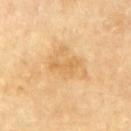Q: Patient demographics?
A: male, aged 83 or over
Q: What lighting was used for the tile?
A: cross-polarized illumination
Q: How large is the lesion?
A: ~3.5 mm (longest diameter)
Q: Where on the body is the lesion?
A: the back
Q: Automated lesion metrics?
A: roughly 8 lightness units darker than nearby skin and a normalized lesion–skin contrast near 5.5
Q: What kind of image is this?
A: ~15 mm tile from a whole-body skin photo